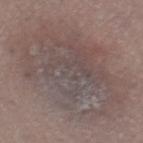Findings:
– follow-up · total-body-photography surveillance lesion; no biopsy
– tile lighting · white-light illumination
– image · 15 mm crop, total-body photography
– lesion diameter · ≈13 mm
– body site · the right thigh
– subject · female, roughly 55 years of age